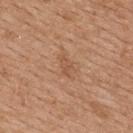biopsy status — total-body-photography surveillance lesion; no biopsy
TBP lesion metrics — border irregularity of about 3.5 on a 0–10 scale, internal color variation of about 2 on a 0–10 scale, and radial color variation of about 0.5; an automated nevus-likeness rating near 0 out of 100
diameter — ≈3 mm
image — ~15 mm tile from a whole-body skin photo
patient — female, approximately 45 years of age
site — the upper back
illumination — white-light illumination